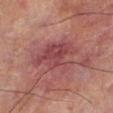Imaged with cross-polarized lighting. Measured at roughly 6.5 mm in maximum diameter. A 15 mm close-up tile from a total-body photography series done for melanoma screening. A male subject aged around 70. Located on the left lower leg.